The total-body-photography lesion software estimated a lesion area of about 13 mm², a shape eccentricity near 0.5, and a shape-asymmetry score of about 0.35 (0 = symmetric). The software also gave an average lesion color of about L≈53 a*≈25 b*≈22 (CIELAB) and a lesion–skin lightness drop of about 10. The software also gave a border-irregularity rating of about 5.5/10, a color-variation rating of about 3.5/10, and radial color variation of about 1. And it measured an automated nevus-likeness rating near 5 out of 100. The recorded lesion diameter is about 4.5 mm. Located on the left thigh. A female subject, approximately 75 years of age. A 15 mm crop from a total-body photograph taken for skin-cancer surveillance — 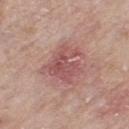Q: What is the histopathologic diagnosis?
A: a superficial basal cell carcinoma — a skin cancer